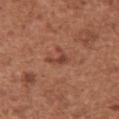{"biopsy_status": "not biopsied; imaged during a skin examination", "lighting": "white-light", "patient": {"sex": "female", "age_approx": 50}, "automated_metrics": {"area_mm2_approx": 2.5, "shape_asymmetry": 0.55, "border_irregularity_0_10": 5.5, "nevus_likeness_0_100": 30, "lesion_detection_confidence_0_100": 100}, "site": "right upper arm", "lesion_size": {"long_diameter_mm_approx": 2.5}, "image": {"source": "total-body photography crop", "field_of_view_mm": 15}}